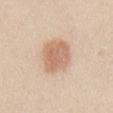Imaged during a routine full-body skin examination; the lesion was not biopsied and no histopathology is available. This is a white-light tile. On the chest. A male subject, aged approximately 25. Longest diameter approximately 5 mm. A 15 mm crop from a total-body photograph taken for skin-cancer surveillance.